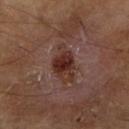This lesion was catalogued during total-body skin photography and was not selected for biopsy. Imaged with cross-polarized lighting. The lesion-visualizer software estimated an area of roughly 9.5 mm², an eccentricity of roughly 0.85, and a symmetry-axis asymmetry near 0.25. It also reported a lesion color around L≈30 a*≈20 b*≈22 in CIELAB, a lesion–skin lightness drop of about 9, and a lesion-to-skin contrast of about 9.5 (normalized; higher = more distinct). The analysis additionally found a border-irregularity index near 3/10 and a peripheral color-asymmetry measure near 1.5. The analysis additionally found a classifier nevus-likeness of about 95/100 and lesion-presence confidence of about 100/100. A region of skin cropped from a whole-body photographic capture, roughly 15 mm wide. A male patient aged 63–67.